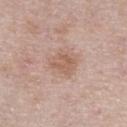biopsy status — catalogued during a skin exam; not biopsied
imaging modality — ~15 mm crop, total-body skin-cancer survey
body site — the abdomen
lesion size — ≈3.5 mm
lighting — white-light
patient — male, aged 53–57
image-analysis metrics — a lesion color around L≈59 a*≈19 b*≈28 in CIELAB, about 8 CIELAB-L* units darker than the surrounding skin, and a lesion-to-skin contrast of about 6 (normalized; higher = more distinct); border irregularity of about 3 on a 0–10 scale and peripheral color asymmetry of about 1; an automated nevus-likeness rating near 5 out of 100 and a detector confidence of about 100 out of 100 that the crop contains a lesion